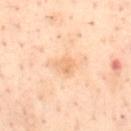Q: Is there a histopathology result?
A: imaged on a skin check; not biopsied
Q: What are the patient's age and sex?
A: female, in their mid- to late 50s
Q: How was this image acquired?
A: ~15 mm tile from a whole-body skin photo
Q: Illumination type?
A: cross-polarized illumination
Q: What is the lesion's diameter?
A: about 2.5 mm
Q: Where on the body is the lesion?
A: the mid back